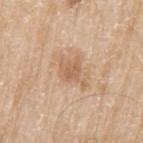About 4 mm across. The lesion is located on the left upper arm. The lesion-visualizer software estimated a footprint of about 6 mm², a shape eccentricity near 0.7, and a symmetry-axis asymmetry near 0.4. The software also gave an average lesion color of about L≈61 a*≈19 b*≈33 (CIELAB), a lesion–skin lightness drop of about 9, and a normalized lesion–skin contrast near 6. The analysis additionally found an automated nevus-likeness rating near 0 out of 100 and a lesion-detection confidence of about 100/100. A male patient, in their 80s. Imaged with white-light lighting. A 15 mm close-up extracted from a 3D total-body photography capture.